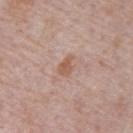<tbp_lesion>
<biopsy_status>not biopsied; imaged during a skin examination</biopsy_status>
<lesion_size>
  <long_diameter_mm_approx>2.5</long_diameter_mm_approx>
</lesion_size>
<patient>
  <sex>male</sex>
  <age_approx>70</age_approx>
</patient>
<image>
  <source>total-body photography crop</source>
  <field_of_view_mm>15</field_of_view_mm>
</image>
<site>abdomen</site>
<lighting>white-light</lighting>
</tbp_lesion>